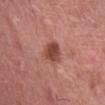{
  "biopsy_status": "not biopsied; imaged during a skin examination",
  "image": {
    "source": "total-body photography crop",
    "field_of_view_mm": 15
  },
  "lighting": "white-light",
  "automated_metrics": {
    "cielab_L": 46,
    "cielab_a": 26,
    "cielab_b": 27,
    "vs_skin_darker_L": 12.0,
    "vs_skin_contrast_norm": 9.0
  },
  "site": "abdomen",
  "lesion_size": {
    "long_diameter_mm_approx": 3.0
  },
  "patient": {
    "sex": "female",
    "age_approx": 60
  }
}